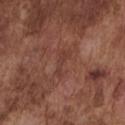Recorded during total-body skin imaging; not selected for excision or biopsy.
A male subject aged around 75.
The lesion is located on the chest.
A close-up tile cropped from a whole-body skin photograph, about 15 mm across.
Measured at roughly 3.5 mm in maximum diameter.